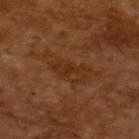Captured during whole-body skin photography for melanoma surveillance; the lesion was not biopsied. Automated tile analysis of the lesion measured a footprint of about 11 mm² and a symmetry-axis asymmetry near 0.4. The software also gave a lesion color around L≈30 a*≈20 b*≈31 in CIELAB, roughly 5 lightness units darker than nearby skin, and a normalized lesion–skin contrast near 5.5. The lesion's longest dimension is about 6.5 mm. Imaged with cross-polarized lighting. The patient is a male roughly 65 years of age. A region of skin cropped from a whole-body photographic capture, roughly 15 mm wide.